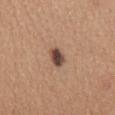No biopsy was performed on this lesion — it was imaged during a full skin examination and was not determined to be concerning. Cropped from a total-body skin-imaging series; the visible field is about 15 mm. The lesion's longest dimension is about 3 mm. From the mid back. A female subject about 35 years old. The total-body-photography lesion software estimated an area of roughly 4.5 mm², an outline eccentricity of about 0.8 (0 = round, 1 = elongated), and a shape-asymmetry score of about 0.15 (0 = symmetric). And it measured a lesion color around L≈46 a*≈18 b*≈26 in CIELAB, about 16 CIELAB-L* units darker than the surrounding skin, and a lesion-to-skin contrast of about 12 (normalized; higher = more distinct). And it measured border irregularity of about 1.5 on a 0–10 scale, a color-variation rating of about 4.5/10, and radial color variation of about 1.5. It also reported a nevus-likeness score of about 90/100.